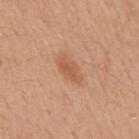Assessment: The lesion was tiled from a total-body skin photograph and was not biopsied. Clinical summary: Measured at roughly 4.5 mm in maximum diameter. Cropped from a total-body skin-imaging series; the visible field is about 15 mm. Captured under white-light illumination. The subject is a male aged approximately 50. The lesion is on the mid back.